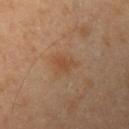Clinical summary:
A male patient in their mid-50s. This is a cross-polarized tile. An algorithmic analysis of the crop reported an eccentricity of roughly 0.75 and two-axis asymmetry of about 0.35. The software also gave roughly 6 lightness units darker than nearby skin and a normalized border contrast of about 5.5. The software also gave a border-irregularity rating of about 3.5/10, a within-lesion color-variation index near 1.5/10, and radial color variation of about 0.5. The lesion is on the left upper arm. This image is a 15 mm lesion crop taken from a total-body photograph. Longest diameter approximately 3 mm.